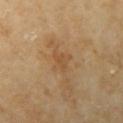– notes · no biopsy performed (imaged during a skin exam)
– anatomic site · the left upper arm
– subject · female, aged approximately 60
– image · total-body-photography crop, ~15 mm field of view